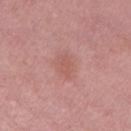{"biopsy_status": "not biopsied; imaged during a skin examination", "site": "left thigh", "image": {"source": "total-body photography crop", "field_of_view_mm": 15}, "automated_metrics": {"area_mm2_approx": 7.5, "eccentricity": 0.7, "border_irregularity_0_10": 2.0, "nevus_likeness_0_100": 0}, "lighting": "white-light", "patient": {"sex": "female", "age_approx": 40}, "lesion_size": {"long_diameter_mm_approx": 3.5}}